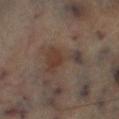follow-up = total-body-photography surveillance lesion; no biopsy | imaging modality = total-body-photography crop, ~15 mm field of view | TBP lesion metrics = a border-irregularity index near 8/10, a within-lesion color-variation index near 3.5/10, and a peripheral color-asymmetry measure near 0.5 | lighting = cross-polarized illumination | anatomic site = the leg | lesion diameter = about 5 mm | subject = male, in their mid- to late 60s.